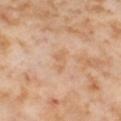Recorded during total-body skin imaging; not selected for excision or biopsy.
The lesion is on the left thigh.
A female subject, approximately 55 years of age.
The recorded lesion diameter is about 3.5 mm.
The tile uses cross-polarized illumination.
A lesion tile, about 15 mm wide, cut from a 3D total-body photograph.
Automated tile analysis of the lesion measured a lesion color around L≈65 a*≈19 b*≈35 in CIELAB, a lesion–skin lightness drop of about 6, and a normalized border contrast of about 5. It also reported border irregularity of about 3.5 on a 0–10 scale, a color-variation rating of about 1.5/10, and radial color variation of about 0.5.